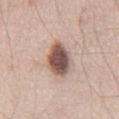Case summary:
- body site · the chest
- patient · male, roughly 55 years of age
- tile lighting · white-light illumination
- automated lesion analysis · an area of roughly 11 mm², an eccentricity of roughly 0.85, and a symmetry-axis asymmetry near 0.2; an average lesion color of about L≈53 a*≈18 b*≈23 (CIELAB) and a normalized lesion–skin contrast near 12; border irregularity of about 2.5 on a 0–10 scale, internal color variation of about 6 on a 0–10 scale, and radial color variation of about 2
- acquisition · ~15 mm crop, total-body skin-cancer survey
- lesion size · ~5.5 mm (longest diameter)